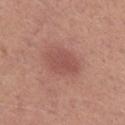Clinical impression:
This lesion was catalogued during total-body skin photography and was not selected for biopsy.
Background:
About 4.5 mm across. This is a white-light tile. The patient is a male aged 73 to 77. The lesion is on the leg. A lesion tile, about 15 mm wide, cut from a 3D total-body photograph.